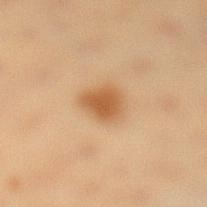This lesion was catalogued during total-body skin photography and was not selected for biopsy.
A female subject, approximately 40 years of age.
Located on the left lower leg.
Cropped from a whole-body photographic skin survey; the tile spans about 15 mm.
About 3.5 mm across.
The tile uses cross-polarized illumination.
The total-body-photography lesion software estimated an average lesion color of about L≈49 a*≈19 b*≈35 (CIELAB), roughly 10 lightness units darker than nearby skin, and a normalized border contrast of about 8.5. It also reported an automated nevus-likeness rating near 100 out of 100.